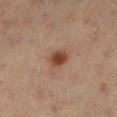Clinical summary: The lesion is located on the left lower leg. A female subject in their mid- to late 50s. This image is a 15 mm lesion crop taken from a total-body photograph.